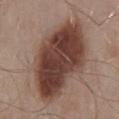{"lesion_size": {"long_diameter_mm_approx": 11.0}, "image": {"source": "total-body photography crop", "field_of_view_mm": 15}, "lighting": "white-light", "site": "mid back", "patient": {"sex": "male", "age_approx": 55}}